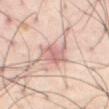notes: no biopsy performed (imaged during a skin exam)
lesion diameter: ~4.5 mm (longest diameter)
acquisition: total-body-photography crop, ~15 mm field of view
automated lesion analysis: a footprint of about 10 mm²; a border-irregularity rating of about 3.5/10 and internal color variation of about 4 on a 0–10 scale
patient: male, in their mid-50s
body site: the abdomen
illumination: cross-polarized illumination